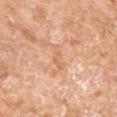{"biopsy_status": "not biopsied; imaged during a skin examination", "automated_metrics": {"area_mm2_approx": 3.5, "shape_asymmetry": 0.4, "vs_skin_darker_L": 7.0, "vs_skin_contrast_norm": 5.0, "color_variation_0_10": 0.5, "peripheral_color_asymmetry": 0.0, "nevus_likeness_0_100": 0, "lesion_detection_confidence_0_100": 95}, "site": "left upper arm", "lighting": "white-light", "lesion_size": {"long_diameter_mm_approx": 3.5}, "patient": {"sex": "female", "age_approx": 75}, "image": {"source": "total-body photography crop", "field_of_view_mm": 15}}